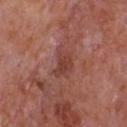No biopsy was performed on this lesion — it was imaged during a full skin examination and was not determined to be concerning. Cropped from a whole-body photographic skin survey; the tile spans about 15 mm. The lesion's longest dimension is about 3.5 mm. The patient is a male aged 63–67. The lesion is located on the chest. The tile uses white-light illumination.